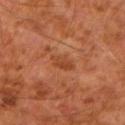Imaged during a routine full-body skin examination; the lesion was not biopsied and no histopathology is available.